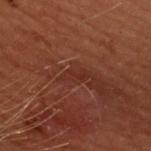{"biopsy_status": "not biopsied; imaged during a skin examination", "site": "upper back", "lesion_size": {"long_diameter_mm_approx": 3.0}, "image": {"source": "total-body photography crop", "field_of_view_mm": 15}, "lighting": "cross-polarized", "patient": {"sex": "female", "age_approx": 50}, "automated_metrics": {"area_mm2_approx": 3.5, "shape_asymmetry": 0.35, "color_variation_0_10": 0.5, "peripheral_color_asymmetry": 0.0}}